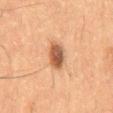No biopsy was performed on this lesion — it was imaged during a full skin examination and was not determined to be concerning. The total-body-photography lesion software estimated an average lesion color of about L≈50 a*≈21 b*≈32 (CIELAB), a lesion–skin lightness drop of about 14, and a normalized lesion–skin contrast near 9.5. The subject is a male aged approximately 60. Imaged with cross-polarized lighting. Longest diameter approximately 4 mm. Cropped from a whole-body photographic skin survey; the tile spans about 15 mm.